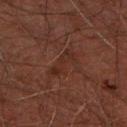follow-up=total-body-photography surveillance lesion; no biopsy | acquisition=~15 mm tile from a whole-body skin photo | automated lesion analysis=lesion-presence confidence of about 95/100 | patient=male, roughly 45 years of age | lesion size=about 4 mm | lighting=cross-polarized illumination | anatomic site=the upper back.